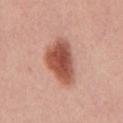{"site": "front of the torso", "lighting": "white-light", "image": {"source": "total-body photography crop", "field_of_view_mm": 15}, "lesion_size": {"long_diameter_mm_approx": 6.0}, "patient": {"sex": "male", "age_approx": 60}}